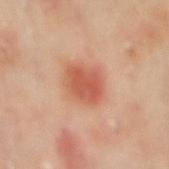acquisition=total-body-photography crop, ~15 mm field of view | body site=the back | patient=male, aged approximately 65 | size=~4 mm (longest diameter).